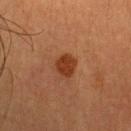The lesion was photographed on a routine skin check and not biopsied; there is no pathology result.
Located on the head or neck.
A lesion tile, about 15 mm wide, cut from a 3D total-body photograph.
The lesion-visualizer software estimated a within-lesion color-variation index near 2/10 and radial color variation of about 1. The analysis additionally found a nevus-likeness score of about 100/100 and lesion-presence confidence of about 100/100.
The subject is a female aged 38 to 42.